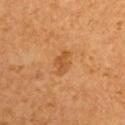Clinical impression: No biopsy was performed on this lesion — it was imaged during a full skin examination and was not determined to be concerning. Clinical summary: The recorded lesion diameter is about 3 mm. The subject is a male aged 53 to 57. Imaged with cross-polarized lighting. From the right upper arm. Cropped from a whole-body photographic skin survey; the tile spans about 15 mm.